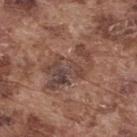| feature | finding |
|---|---|
| TBP lesion metrics | a shape eccentricity near 0.85; a lesion color around L≈44 a*≈18 b*≈23 in CIELAB and a normalized border contrast of about 7.5 |
| subject | male, in their mid-70s |
| body site | the upper back |
| illumination | white-light illumination |
| lesion diameter | ~7 mm (longest diameter) |
| image | total-body-photography crop, ~15 mm field of view |